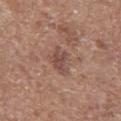biopsy status — catalogued during a skin exam; not biopsied
image-analysis metrics — an average lesion color of about L≈48 a*≈19 b*≈24 (CIELAB), about 9 CIELAB-L* units darker than the surrounding skin, and a lesion-to-skin contrast of about 6.5 (normalized; higher = more distinct); a border-irregularity index near 3.5/10 and a peripheral color-asymmetry measure near 1
lighting — white-light
body site — the leg
image — total-body-photography crop, ~15 mm field of view
subject — female, in their mid-70s